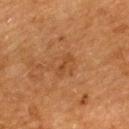Image and clinical context:
From the upper back. This image is a 15 mm lesion crop taken from a total-body photograph. Automated tile analysis of the lesion measured an area of roughly 2 mm², an eccentricity of roughly 0.95, and two-axis asymmetry of about 0.45. And it measured a classifier nevus-likeness of about 0/100 and a detector confidence of about 100 out of 100 that the crop contains a lesion. A female patient aged 53–57. The tile uses cross-polarized illumination. Measured at roughly 2.5 mm in maximum diameter.